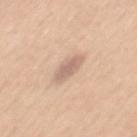Part of a total-body skin-imaging series; this lesion was reviewed on a skin check and was not flagged for biopsy.
The lesion-visualizer software estimated a shape eccentricity near 0.9 and a symmetry-axis asymmetry near 0.2. And it measured a mean CIELAB color near L≈65 a*≈17 b*≈27 and about 10 CIELAB-L* units darker than the surrounding skin. The software also gave radial color variation of about 0.5.
A male subject, aged around 50.
From the back.
Cropped from a whole-body photographic skin survey; the tile spans about 15 mm.
The lesion's longest dimension is about 4 mm.